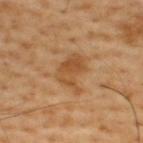size = about 4.5 mm; body site = the back; imaging modality = total-body-photography crop, ~15 mm field of view; patient = male, in their mid-50s.